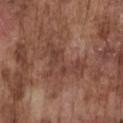Assessment:
This lesion was catalogued during total-body skin photography and was not selected for biopsy.
Image and clinical context:
From the chest. The recorded lesion diameter is about 6.5 mm. A 15 mm close-up tile from a total-body photography series done for melanoma screening. A male patient, roughly 75 years of age.